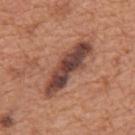Q: Was this lesion biopsied?
A: no biopsy performed (imaged during a skin exam)